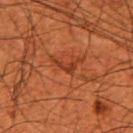Q: How was this image acquired?
A: total-body-photography crop, ~15 mm field of view
Q: Where on the body is the lesion?
A: the arm
Q: What are the patient's age and sex?
A: male, aged approximately 50
Q: What lighting was used for the tile?
A: cross-polarized illumination
Q: Automated lesion metrics?
A: an area of roughly 2.5 mm²; a normalized lesion–skin contrast near 6.5; border irregularity of about 5.5 on a 0–10 scale, internal color variation of about 0 on a 0–10 scale, and radial color variation of about 0